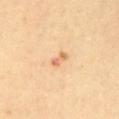Background:
Automated image analysis of the tile measured an average lesion color of about L≈71 a*≈24 b*≈40 (CIELAB), a lesion–skin lightness drop of about 11, and a lesion-to-skin contrast of about 6.5 (normalized; higher = more distinct). The analysis additionally found a within-lesion color-variation index near 0/10 and radial color variation of about 0. This image is a 15 mm lesion crop taken from a total-body photograph. From the mid back. About 2.5 mm across. A female patient in their 40s.